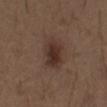The lesion was tiled from a total-body skin photograph and was not biopsied.
This image is a 15 mm lesion crop taken from a total-body photograph.
The subject is a male about 50 years old.
Imaged with white-light lighting.
Located on the abdomen.